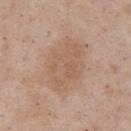Imaged during a routine full-body skin examination; the lesion was not biopsied and no histopathology is available. A lesion tile, about 15 mm wide, cut from a 3D total-body photograph. From the chest. The patient is a male aged around 55. Captured under white-light illumination.